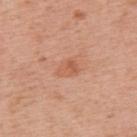| feature | finding |
|---|---|
| workup | no biopsy performed (imaged during a skin exam) |
| subject | male, approximately 60 years of age |
| body site | the upper back |
| image source | total-body-photography crop, ~15 mm field of view |
| lighting | white-light illumination |
| automated metrics | a footprint of about 4.5 mm², a shape eccentricity near 0.7, and two-axis asymmetry of about 0.3 |
| lesion diameter | about 2.5 mm |